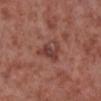| feature | finding |
|---|---|
| follow-up | imaged on a skin check; not biopsied |
| imaging modality | ~15 mm crop, total-body skin-cancer survey |
| tile lighting | white-light illumination |
| anatomic site | the right lower leg |
| lesion size | ≈4 mm |
| subject | male, aged approximately 55 |